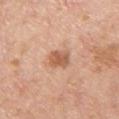<record>
  <biopsy_status>not biopsied; imaged during a skin examination</biopsy_status>
  <patient>
    <sex>male</sex>
    <age_approx>60</age_approx>
  </patient>
  <lighting>white-light</lighting>
  <site>upper back</site>
  <automated_metrics>
    <area_mm2_approx>5.0</area_mm2_approx>
    <eccentricity>0.7</eccentricity>
    <shape_asymmetry>0.15</shape_asymmetry>
    <lesion_detection_confidence_0_100>100</lesion_detection_confidence_0_100>
  </automated_metrics>
  <image>
    <source>total-body photography crop</source>
    <field_of_view_mm>15</field_of_view_mm>
  </image>
</record>